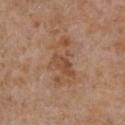<case>
  <biopsy_status>not biopsied; imaged during a skin examination</biopsy_status>
  <lesion_size>
    <long_diameter_mm_approx>6.0</long_diameter_mm_approx>
  </lesion_size>
  <patient>
    <sex>female</sex>
    <age_approx>75</age_approx>
  </patient>
  <image>
    <source>total-body photography crop</source>
    <field_of_view_mm>15</field_of_view_mm>
  </image>
  <lighting>white-light</lighting>
  <site>chest</site>
  <automated_metrics>
    <area_mm2_approx>14.0</area_mm2_approx>
    <shape_asymmetry>0.4</shape_asymmetry>
    <border_irregularity_0_10>5.5</border_irregularity_0_10>
    <color_variation_0_10>5.0</color_variation_0_10>
    <peripheral_color_asymmetry>1.5</peripheral_color_asymmetry>
  </automated_metrics>
</case>